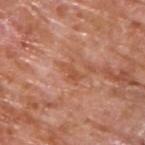follow-up = no biopsy performed (imaged during a skin exam) | site = the upper back | acquisition = ~15 mm tile from a whole-body skin photo | patient = male, aged around 65 | lighting = white-light illumination | lesion size = ≈2.5 mm.